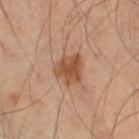Q: Is there a histopathology result?
A: total-body-photography surveillance lesion; no biopsy
Q: Lesion location?
A: the left thigh
Q: Patient demographics?
A: male, aged around 55
Q: How large is the lesion?
A: ≈3.5 mm
Q: What is the imaging modality?
A: ~15 mm tile from a whole-body skin photo